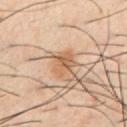The lesion was tiled from a total-body skin photograph and was not biopsied. On the chest. The patient is a male aged 38–42. This is a cross-polarized tile. Cropped from a total-body skin-imaging series; the visible field is about 15 mm. Longest diameter approximately 3 mm.